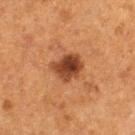The lesion was photographed on a routine skin check and not biopsied; there is no pathology result. Cropped from a total-body skin-imaging series; the visible field is about 15 mm. A female subject roughly 50 years of age. The lesion is located on the leg. About 3.5 mm across.